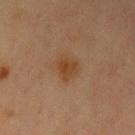Captured during whole-body skin photography for melanoma surveillance; the lesion was not biopsied. A female subject roughly 65 years of age. The lesion is located on the left upper arm. A 15 mm crop from a total-body photograph taken for skin-cancer surveillance.